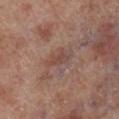biopsy status: catalogued during a skin exam; not biopsied